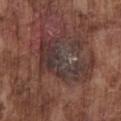Recorded during total-body skin imaging; not selected for excision or biopsy. Cropped from a whole-body photographic skin survey; the tile spans about 15 mm. Automated tile analysis of the lesion measured a mean CIELAB color near L≈34 a*≈17 b*≈18, roughly 8 lightness units darker than nearby skin, and a lesion-to-skin contrast of about 8 (normalized; higher = more distinct). The software also gave a border-irregularity index near 10/10 and radial color variation of about 2. The subject is a male aged approximately 75. Located on the chest. The recorded lesion diameter is about 7.5 mm.